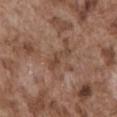| field | value |
|---|---|
| notes | total-body-photography surveillance lesion; no biopsy |